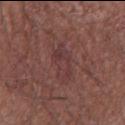Imaged during a routine full-body skin examination; the lesion was not biopsied and no histopathology is available. From the right forearm. A 15 mm close-up tile from a total-body photography series done for melanoma screening. The total-body-photography lesion software estimated a lesion color around L≈37 a*≈22 b*≈18 in CIELAB, a lesion–skin lightness drop of about 5, and a normalized lesion–skin contrast near 5. It also reported an automated nevus-likeness rating near 0 out of 100 and a lesion-detection confidence of about 100/100. The patient is a male in their mid-60s. Imaged with white-light lighting.